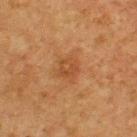The lesion was tiled from a total-body skin photograph and was not biopsied. Located on the upper back. The subject is a male aged 73–77. A region of skin cropped from a whole-body photographic capture, roughly 15 mm wide.